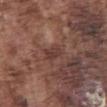No biopsy was performed on this lesion — it was imaged during a full skin examination and was not determined to be concerning. Approximately 3 mm at its widest. A male subject about 75 years old. A 15 mm close-up extracted from a 3D total-body photography capture. The lesion-visualizer software estimated a lesion–skin lightness drop of about 7 and a normalized border contrast of about 6.5. It also reported a color-variation rating of about 3/10. The software also gave an automated nevus-likeness rating near 0 out of 100. Captured under white-light illumination. On the abdomen.